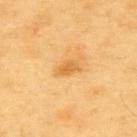Imaged during a routine full-body skin examination; the lesion was not biopsied and no histopathology is available. The recorded lesion diameter is about 3 mm. The total-body-photography lesion software estimated an outline eccentricity of about 0.85 (0 = round, 1 = elongated) and a shape-asymmetry score of about 0.3 (0 = symmetric). The analysis additionally found border irregularity of about 3 on a 0–10 scale, internal color variation of about 2 on a 0–10 scale, and radial color variation of about 1. The software also gave an automated nevus-likeness rating near 0 out of 100 and a detector confidence of about 100 out of 100 that the crop contains a lesion. Located on the upper back. A 15 mm close-up tile from a total-body photography series done for melanoma screening. A male subject approximately 60 years of age.